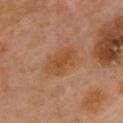Findings:
* biopsy status: total-body-photography surveillance lesion; no biopsy
* image-analysis metrics: a footprint of about 11 mm², a shape eccentricity near 0.8, and a shape-asymmetry score of about 0.15 (0 = symmetric); a border-irregularity rating of about 2/10, a within-lesion color-variation index near 3/10, and radial color variation of about 1
* patient: female, in their 60s
* lighting: cross-polarized
* imaging modality: ~15 mm tile from a whole-body skin photo
* location: the chest
* lesion size: ≈5 mm